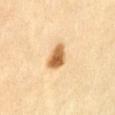The subject is a female aged around 60.
The lesion is on the abdomen.
Captured under cross-polarized illumination.
Cropped from a whole-body photographic skin survey; the tile spans about 15 mm.